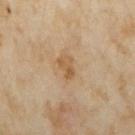Captured under cross-polarized illumination. From the right upper arm. A 15 mm crop from a total-body photograph taken for skin-cancer surveillance. The subject is a female aged around 35. The lesion's longest dimension is about 2.5 mm.